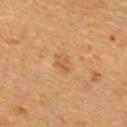Part of a total-body skin-imaging series; this lesion was reviewed on a skin check and was not flagged for biopsy. A subject aged 58 to 62. Longest diameter approximately 2.5 mm. Automated image analysis of the tile measured a detector confidence of about 100 out of 100 that the crop contains a lesion. The tile uses cross-polarized illumination. From the back. A close-up tile cropped from a whole-body skin photograph, about 15 mm across.